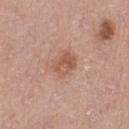Clinical impression:
This lesion was catalogued during total-body skin photography and was not selected for biopsy.